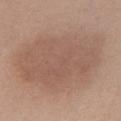This is a white-light tile.
The lesion is located on the chest.
A 15 mm crop from a total-body photograph taken for skin-cancer surveillance.
A female patient aged 58–62.
Automated image analysis of the tile measured a mean CIELAB color near L≈56 a*≈18 b*≈27 and a normalized lesion–skin contrast near 5.5. The analysis additionally found a border-irregularity rating of about 2.5/10 and internal color variation of about 3 on a 0–10 scale. The software also gave a classifier nevus-likeness of about 75/100 and a detector confidence of about 100 out of 100 that the crop contains a lesion.
Approximately 13 mm at its widest.